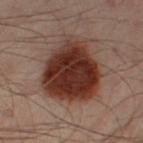Imaged during a routine full-body skin examination; the lesion was not biopsied and no histopathology is available. On the left leg. The total-body-photography lesion software estimated an average lesion color of about L≈26 a*≈18 b*≈21 (CIELAB) and a normalized border contrast of about 13.5. The software also gave a border-irregularity rating of about 2.5/10 and a color-variation rating of about 6/10. A lesion tile, about 15 mm wide, cut from a 3D total-body photograph. About 8 mm across. The subject is a male roughly 50 years of age. The tile uses cross-polarized illumination.